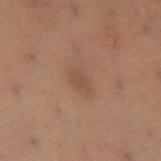Recorded during total-body skin imaging; not selected for excision or biopsy.
From the left thigh.
A female patient in their mid-50s.
Measured at roughly 2.5 mm in maximum diameter.
Automated tile analysis of the lesion measured a mean CIELAB color near L≈39 a*≈17 b*≈24 and a normalized border contrast of about 5. It also reported a border-irregularity index near 2.5/10, internal color variation of about 1.5 on a 0–10 scale, and radial color variation of about 0.5.
A 15 mm close-up tile from a total-body photography series done for melanoma screening.
Imaged with cross-polarized lighting.